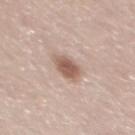Acquisition and patient details:
Automated image analysis of the tile measured an area of roughly 6 mm² and a shape eccentricity near 0.6. Located on the upper back. Approximately 3 mm at its widest. A region of skin cropped from a whole-body photographic capture, roughly 15 mm wide. This is a white-light tile. A male subject, approximately 60 years of age.